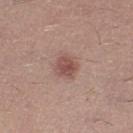• biopsy status: total-body-photography surveillance lesion; no biopsy
• lesion diameter: ≈3 mm
• anatomic site: the left lower leg
• image source: 15 mm crop, total-body photography
• patient: male, roughly 25 years of age
• tile lighting: white-light illumination